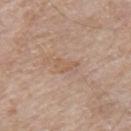  biopsy_status: not biopsied; imaged during a skin examination
  lesion_size:
    long_diameter_mm_approx: 2.5
  lighting: white-light
  image:
    source: total-body photography crop
    field_of_view_mm: 15
  site: mid back
  patient:
    sex: male
    age_approx: 65